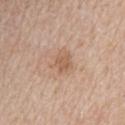Captured during whole-body skin photography for melanoma surveillance; the lesion was not biopsied.
A female subject, in their mid-40s.
Located on the upper back.
A region of skin cropped from a whole-body photographic capture, roughly 15 mm wide.
The recorded lesion diameter is about 3 mm.
An algorithmic analysis of the crop reported a footprint of about 6 mm², an eccentricity of roughly 0.5, and a symmetry-axis asymmetry near 0.35. And it measured a border-irregularity rating of about 4/10, a color-variation rating of about 2/10, and radial color variation of about 0.5.